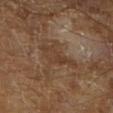Impression:
Recorded during total-body skin imaging; not selected for excision or biopsy.
Image and clinical context:
This image is a 15 mm lesion crop taken from a total-body photograph. The subject is a male approximately 65 years of age. Captured under cross-polarized illumination.